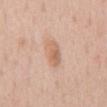Clinical impression: Recorded during total-body skin imaging; not selected for excision or biopsy. Acquisition and patient details: Cropped from a whole-body photographic skin survey; the tile spans about 15 mm. The subject is a male in their mid-50s. Imaged with white-light lighting. Measured at roughly 4 mm in maximum diameter. On the chest. The lesion-visualizer software estimated a lesion color around L≈64 a*≈20 b*≈31 in CIELAB and a normalized lesion–skin contrast near 6. It also reported an automated nevus-likeness rating near 45 out of 100 and a detector confidence of about 100 out of 100 that the crop contains a lesion.